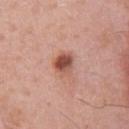No biopsy was performed on this lesion — it was imaged during a full skin examination and was not determined to be concerning.
A male patient, approximately 60 years of age.
A region of skin cropped from a whole-body photographic capture, roughly 15 mm wide.
Longest diameter approximately 3 mm.
On the chest.
The tile uses white-light illumination.